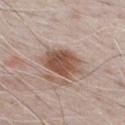Notes:
* biopsy status — imaged on a skin check; not biopsied